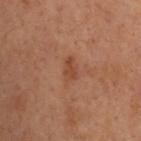Captured during whole-body skin photography for melanoma surveillance; the lesion was not biopsied. On the upper back. This image is a 15 mm lesion crop taken from a total-body photograph. The total-body-photography lesion software estimated a classifier nevus-likeness of about 0/100 and a lesion-detection confidence of about 100/100. The recorded lesion diameter is about 3 mm. The patient is a female aged 53 to 57.